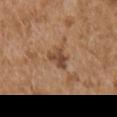| feature | finding |
|---|---|
| biopsy status | catalogued during a skin exam; not biopsied |
| acquisition | ~15 mm crop, total-body skin-cancer survey |
| lesion size | about 3.5 mm |
| tile lighting | white-light illumination |
| subject | male, aged 73 to 77 |
| location | the right upper arm |
| automated lesion analysis | an area of roughly 4.5 mm², a shape eccentricity near 0.75, and a shape-asymmetry score of about 0.45 (0 = symmetric); a lesion-detection confidence of about 100/100 |